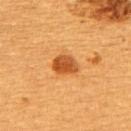Image and clinical context: A female patient aged 53 to 57. From the back. A region of skin cropped from a whole-body photographic capture, roughly 15 mm wide.